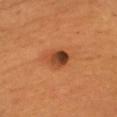Part of a total-body skin-imaging series; this lesion was reviewed on a skin check and was not flagged for biopsy. A lesion tile, about 15 mm wide, cut from a 3D total-body photograph. Imaged with cross-polarized lighting. The patient is a male aged 63–67. Longest diameter approximately 3 mm. From the chest. Automated image analysis of the tile measured internal color variation of about 10 on a 0–10 scale and peripheral color asymmetry of about 4.5.